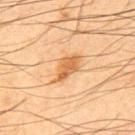biopsy status: imaged on a skin check; not biopsied | location: the mid back | acquisition: 15 mm crop, total-body photography | subject: male, in their mid-40s.